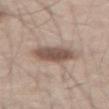This lesion was catalogued during total-body skin photography and was not selected for biopsy. A male subject, aged 58 to 62. Automated tile analysis of the lesion measured a lesion–skin lightness drop of about 13 and a lesion-to-skin contrast of about 9 (normalized; higher = more distinct). The analysis additionally found a within-lesion color-variation index near 4/10 and peripheral color asymmetry of about 1. The analysis additionally found an automated nevus-likeness rating near 90 out of 100. From the leg. Imaged with white-light lighting. A roughly 15 mm field-of-view crop from a total-body skin photograph.